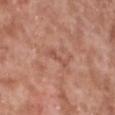This lesion was catalogued during total-body skin photography and was not selected for biopsy.
Measured at roughly 3.5 mm in maximum diameter.
The tile uses white-light illumination.
The subject is a male roughly 55 years of age.
The total-body-photography lesion software estimated an area of roughly 3 mm², a shape eccentricity near 0.95, and two-axis asymmetry of about 0.45.
Cropped from a total-body skin-imaging series; the visible field is about 15 mm.
From the left lower leg.